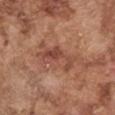biopsy status — no biopsy performed (imaged during a skin exam) | illumination — white-light illumination | diameter — ≈5 mm | subject — male, approximately 75 years of age | location — the left upper arm | imaging modality — ~15 mm crop, total-body skin-cancer survey | TBP lesion metrics — a mean CIELAB color near L≈47 a*≈24 b*≈29, a lesion–skin lightness drop of about 9, and a normalized lesion–skin contrast near 7; a within-lesion color-variation index near 3.5/10 and a peripheral color-asymmetry measure near 1.5; a nevus-likeness score of about 10/100 and a lesion-detection confidence of about 100/100.